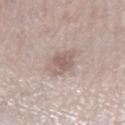Context: The recorded lesion diameter is about 3.5 mm. The patient is a male in their mid- to late 60s. A 15 mm close-up tile from a total-body photography series done for melanoma screening. The tile uses white-light illumination. Located on the right lower leg.